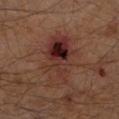From the left lower leg. Imaged with cross-polarized lighting. A male patient, aged 58–62. An algorithmic analysis of the crop reported a footprint of about 23 mm², a shape eccentricity near 0.85, and a shape-asymmetry score of about 0.25 (0 = symmetric). The analysis additionally found roughly 8 lightness units darker than nearby skin and a normalized border contrast of about 8.5. It also reported a border-irregularity rating of about 3.5/10, a color-variation rating of about 10/10, and peripheral color asymmetry of about 5. The lesion's longest dimension is about 7 mm. Cropped from a total-body skin-imaging series; the visible field is about 15 mm.